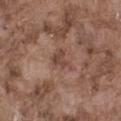workup: no biopsy performed (imaged during a skin exam) | patient: male, about 75 years old | image: ~15 mm crop, total-body skin-cancer survey | size: ≈3 mm | location: the left thigh | TBP lesion metrics: an average lesion color of about L≈45 a*≈19 b*≈25 (CIELAB) and a lesion–skin lightness drop of about 8.